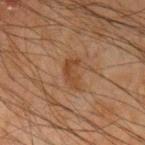notes: no biopsy performed (imaged during a skin exam)
patient: male, approximately 65 years of age
lesion size: ~3.5 mm (longest diameter)
automated metrics: a shape-asymmetry score of about 0.35 (0 = symmetric); a mean CIELAB color near L≈35 a*≈18 b*≈28, a lesion–skin lightness drop of about 6, and a normalized lesion–skin contrast near 6.5
location: the left upper arm
image source: 15 mm crop, total-body photography